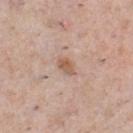Assessment: Imaged during a routine full-body skin examination; the lesion was not biopsied and no histopathology is available. Image and clinical context: A male patient approximately 40 years of age. Automated tile analysis of the lesion measured an average lesion color of about L≈58 a*≈19 b*≈30 (CIELAB), about 9 CIELAB-L* units darker than the surrounding skin, and a normalized border contrast of about 7. And it measured border irregularity of about 2 on a 0–10 scale and a within-lesion color-variation index near 2.5/10. It also reported an automated nevus-likeness rating near 40 out of 100 and a lesion-detection confidence of about 100/100. A lesion tile, about 15 mm wide, cut from a 3D total-body photograph. The lesion's longest dimension is about 2.5 mm. Captured under white-light illumination. On the chest.